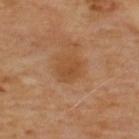The lesion was tiled from a total-body skin photograph and was not biopsied. Measured at roughly 3 mm in maximum diameter. Captured under cross-polarized illumination. On the back. A male subject, roughly 70 years of age. Cropped from a total-body skin-imaging series; the visible field is about 15 mm. Automated tile analysis of the lesion measured an area of roughly 6.5 mm², a shape eccentricity near 0.55, and a shape-asymmetry score of about 0.15 (0 = symmetric). The software also gave a mean CIELAB color near L≈46 a*≈21 b*≈37 and a lesion-to-skin contrast of about 6 (normalized; higher = more distinct).